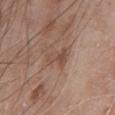Impression: Recorded during total-body skin imaging; not selected for excision or biopsy. Clinical summary: A male subject, roughly 80 years of age. From the chest. Captured under white-light illumination. The total-body-photography lesion software estimated a lesion color around L≈49 a*≈19 b*≈27 in CIELAB, about 7 CIELAB-L* units darker than the surrounding skin, and a normalized lesion–skin contrast near 5.5. It also reported a border-irregularity index near 5/10 and a peripheral color-asymmetry measure near 2. The lesion's longest dimension is about 3.5 mm. A close-up tile cropped from a whole-body skin photograph, about 15 mm across.